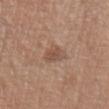The lesion was tiled from a total-body skin photograph and was not biopsied. The lesion is on the chest. An algorithmic analysis of the crop reported a footprint of about 5.5 mm² and a symmetry-axis asymmetry near 0.3. A 15 mm crop from a total-body photograph taken for skin-cancer surveillance. The subject is a female in their mid- to late 50s. The tile uses white-light illumination. The lesion's longest dimension is about 3 mm.